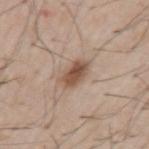Q: Was this lesion biopsied?
A: no biopsy performed (imaged during a skin exam)
Q: Who is the patient?
A: male, roughly 55 years of age
Q: What is the imaging modality?
A: 15 mm crop, total-body photography